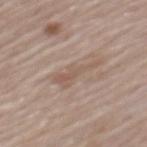The tile uses white-light illumination. Located on the mid back. Automated tile analysis of the lesion measured an area of roughly 5.5 mm², a shape eccentricity near 0.95, and a symmetry-axis asymmetry near 0.55. The analysis additionally found about 7 CIELAB-L* units darker than the surrounding skin and a normalized lesion–skin contrast near 5. The analysis additionally found border irregularity of about 7.5 on a 0–10 scale, a within-lesion color-variation index near 1.5/10, and radial color variation of about 0.5. The software also gave an automated nevus-likeness rating near 0 out of 100 and a lesion-detection confidence of about 80/100. Cropped from a whole-body photographic skin survey; the tile spans about 15 mm. The subject is a male in their 80s.